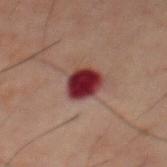Acquisition and patient details:
The lesion is located on the front of the torso. Captured under cross-polarized illumination. A lesion tile, about 15 mm wide, cut from a 3D total-body photograph. A male patient aged approximately 60. The lesion's longest dimension is about 3.5 mm.